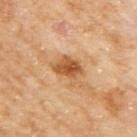Findings:
- workup: catalogued during a skin exam; not biopsied
- lighting: cross-polarized illumination
- acquisition: 15 mm crop, total-body photography
- anatomic site: the right upper arm
- patient: female, approximately 60 years of age